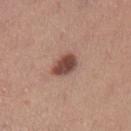Recorded during total-body skin imaging; not selected for excision or biopsy. A female patient, in their mid-40s. From the left lower leg. The tile uses white-light illumination. Longest diameter approximately 3.5 mm. A 15 mm close-up extracted from a 3D total-body photography capture.